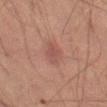No biopsy was performed on this lesion — it was imaged during a full skin examination and was not determined to be concerning. Cropped from a whole-body photographic skin survey; the tile spans about 15 mm. A male patient, aged approximately 70. An algorithmic analysis of the crop reported a footprint of about 4.5 mm², an outline eccentricity of about 0.7 (0 = round, 1 = elongated), and two-axis asymmetry of about 0.3. The analysis additionally found a border-irregularity rating of about 3/10 and internal color variation of about 1 on a 0–10 scale. The analysis additionally found an automated nevus-likeness rating near 5 out of 100 and a lesion-detection confidence of about 100/100. Imaged with cross-polarized lighting. The recorded lesion diameter is about 3 mm. The lesion is located on the right thigh.